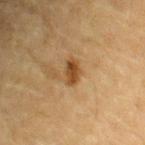• biopsy status: catalogued during a skin exam; not biopsied
• acquisition: ~15 mm crop, total-body skin-cancer survey
• size: ≈3 mm
• body site: the right upper arm
• lighting: cross-polarized
• patient: male, in their mid-80s or older